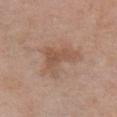Assessment: Imaged during a routine full-body skin examination; the lesion was not biopsied and no histopathology is available. Background: Cropped from a total-body skin-imaging series; the visible field is about 15 mm. Located on the chest. A female subject aged around 60. Imaged with white-light lighting. Measured at roughly 4.5 mm in maximum diameter.